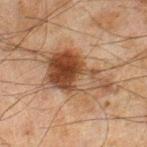Recorded during total-body skin imaging; not selected for excision or biopsy.
The subject is a male roughly 45 years of age.
Located on the right lower leg.
Automated tile analysis of the lesion measured a lesion area of about 21 mm², an outline eccentricity of about 0.85 (0 = round, 1 = elongated), and two-axis asymmetry of about 0.4.
This image is a 15 mm lesion crop taken from a total-body photograph.